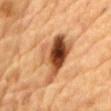workup — total-body-photography surveillance lesion; no biopsy
body site — the chest
acquisition — ~15 mm crop, total-body skin-cancer survey
lesion diameter — ≈7 mm
patient — male, aged 83 or over
TBP lesion metrics — a lesion color around L≈43 a*≈22 b*≈33 in CIELAB, a lesion–skin lightness drop of about 17, and a normalized lesion–skin contrast near 12.5; a border-irregularity rating of about 4/10 and a within-lesion color-variation index near 10/10; a classifier nevus-likeness of about 30/100 and lesion-presence confidence of about 100/100